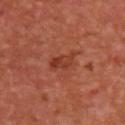Impression:
The lesion was tiled from a total-body skin photograph and was not biopsied.
Acquisition and patient details:
From the upper back. The subject is a male aged 48 to 52. A region of skin cropped from a whole-body photographic capture, roughly 15 mm wide.